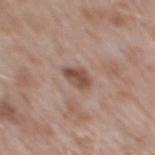The lesion is located on the upper back. A male patient, aged 48 to 52. A close-up tile cropped from a whole-body skin photograph, about 15 mm across.